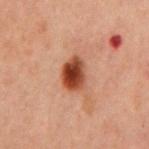Recorded during total-body skin imaging; not selected for excision or biopsy. The tile uses cross-polarized illumination. A male patient, about 60 years old. Approximately 3.5 mm at its widest. The lesion is on the front of the torso. Automated tile analysis of the lesion measured a shape eccentricity near 0.6 and two-axis asymmetry of about 0.2. The software also gave lesion-presence confidence of about 100/100. A close-up tile cropped from a whole-body skin photograph, about 15 mm across.